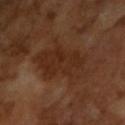Assessment: This lesion was catalogued during total-body skin photography and was not selected for biopsy. Clinical summary: A roughly 15 mm field-of-view crop from a total-body skin photograph. Imaged with cross-polarized lighting. The recorded lesion diameter is about 7.5 mm. The patient is a male in their mid-60s.